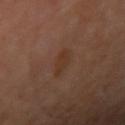Captured during whole-body skin photography for melanoma surveillance; the lesion was not biopsied. Automated image analysis of the tile measured an eccentricity of roughly 0.75 and a shape-asymmetry score of about 0.2 (0 = symmetric). The analysis additionally found a mean CIELAB color near L≈32 a*≈18 b*≈26, about 5 CIELAB-L* units darker than the surrounding skin, and a lesion-to-skin contrast of about 5.5 (normalized; higher = more distinct). The software also gave an automated nevus-likeness rating near 50 out of 100. On the right upper arm. A male subject, aged 58 to 62. The tile uses cross-polarized illumination. About 2.5 mm across. A lesion tile, about 15 mm wide, cut from a 3D total-body photograph.